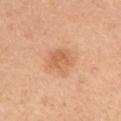| field | value |
|---|---|
| follow-up | no biopsy performed (imaged during a skin exam) |
| tile lighting | white-light |
| subject | female, in their mid- to late 40s |
| automated lesion analysis | an area of roughly 8 mm², an eccentricity of roughly 0.55, and two-axis asymmetry of about 0.25; a lesion color around L≈63 a*≈24 b*≈38 in CIELAB, a lesion–skin lightness drop of about 9, and a normalized border contrast of about 6; an automated nevus-likeness rating near 30 out of 100 and a detector confidence of about 100 out of 100 that the crop contains a lesion |
| size | ≈3.5 mm |
| location | the left upper arm |
| acquisition | 15 mm crop, total-body photography |